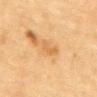Part of a total-body skin-imaging series; this lesion was reviewed on a skin check and was not flagged for biopsy. From the upper back. A roughly 15 mm field-of-view crop from a total-body skin photograph. A female subject aged 78–82. The tile uses cross-polarized illumination. The total-body-photography lesion software estimated an area of roughly 4 mm² and two-axis asymmetry of about 0.45. The analysis additionally found an average lesion color of about L≈56 a*≈19 b*≈40 (CIELAB), a lesion–skin lightness drop of about 7, and a normalized lesion–skin contrast near 5.5.